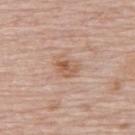Findings:
– patient: female, aged around 65
– anatomic site: the upper back
– automated lesion analysis: a lesion area of about 5 mm², an eccentricity of roughly 0.65, and a shape-asymmetry score of about 0.3 (0 = symmetric); an average lesion color of about L≈58 a*≈20 b*≈30 (CIELAB), a lesion–skin lightness drop of about 9, and a normalized border contrast of about 6.5
– lesion size: about 3 mm
– image: 15 mm crop, total-body photography
– illumination: white-light